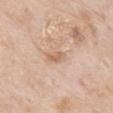Part of a total-body skin-imaging series; this lesion was reviewed on a skin check and was not flagged for biopsy. The tile uses white-light illumination. A lesion tile, about 15 mm wide, cut from a 3D total-body photograph. Approximately 2.5 mm at its widest. Located on the mid back. A male subject, approximately 85 years of age or older.